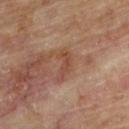workup — total-body-photography surveillance lesion; no biopsy | acquisition — ~15 mm crop, total-body skin-cancer survey | lighting — cross-polarized | anatomic site — the upper back | diameter — ≈4 mm | patient — male, aged approximately 85 or older.